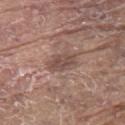| key | value |
|---|---|
| workup | catalogued during a skin exam; not biopsied |
| body site | the upper back |
| image source | 15 mm crop, total-body photography |
| lighting | white-light |
| size | ~3.5 mm (longest diameter) |
| image-analysis metrics | a lesion area of about 5 mm², an eccentricity of roughly 0.85, and a symmetry-axis asymmetry near 0.2; a classifier nevus-likeness of about 0/100 |
| subject | male, aged around 80 |